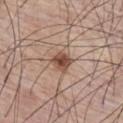{
  "biopsy_status": "not biopsied; imaged during a skin examination",
  "automated_metrics": {
    "area_mm2_approx": 5.0,
    "eccentricity": 0.45,
    "shape_asymmetry": 0.25,
    "border_irregularity_0_10": 2.5,
    "color_variation_0_10": 3.0,
    "peripheral_color_asymmetry": 1.0
  },
  "site": "chest",
  "image": {
    "source": "total-body photography crop",
    "field_of_view_mm": 15
  },
  "patient": {
    "sex": "male",
    "age_approx": 75
  }
}